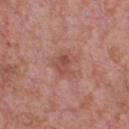Part of a total-body skin-imaging series; this lesion was reviewed on a skin check and was not flagged for biopsy.
The subject is a male roughly 60 years of age.
Located on the chest.
A roughly 15 mm field-of-view crop from a total-body skin photograph.